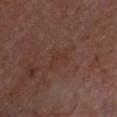Clinical impression: No biopsy was performed on this lesion — it was imaged during a full skin examination and was not determined to be concerning. Context: Located on the head or neck. A patient aged 63–67. The recorded lesion diameter is about 2.5 mm. Imaged with cross-polarized lighting. A close-up tile cropped from a whole-body skin photograph, about 15 mm across. An algorithmic analysis of the crop reported a footprint of about 3 mm², an outline eccentricity of about 0.85 (0 = round, 1 = elongated), and a symmetry-axis asymmetry near 0.55. The software also gave a lesion color around L≈32 a*≈19 b*≈24 in CIELAB and a lesion-to-skin contrast of about 5 (normalized; higher = more distinct). And it measured a detector confidence of about 100 out of 100 that the crop contains a lesion.